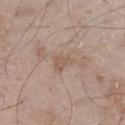{
  "biopsy_status": "not biopsied; imaged during a skin examination",
  "lesion_size": {
    "long_diameter_mm_approx": 2.5
  },
  "image": {
    "source": "total-body photography crop",
    "field_of_view_mm": 15
  },
  "patient": {
    "sex": "male",
    "age_approx": 65
  },
  "lighting": "white-light",
  "site": "left thigh"
}